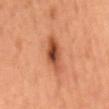| feature | finding |
|---|---|
| biopsy status | imaged on a skin check; not biopsied |
| subject | female |
| image source | 15 mm crop, total-body photography |
| site | the mid back |